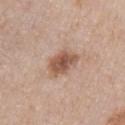{"automated_metrics": {"border_irregularity_0_10": 2.5, "color_variation_0_10": 4.0}, "image": {"source": "total-body photography crop", "field_of_view_mm": 15}, "lighting": "white-light", "lesion_size": {"long_diameter_mm_approx": 4.0}, "site": "right upper arm", "patient": {"sex": "female", "age_approx": 65}}